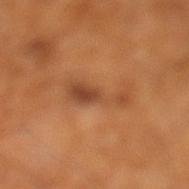| field | value |
|---|---|
| follow-up | catalogued during a skin exam; not biopsied |
| site | the leg |
| size | about 5.5 mm |
| TBP lesion metrics | a border-irregularity index near 7.5/10, a color-variation rating of about 3/10, and radial color variation of about 1 |
| illumination | cross-polarized illumination |
| subject | male, aged approximately 65 |
| imaging modality | ~15 mm tile from a whole-body skin photo |